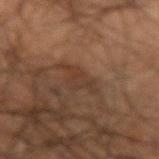Clinical impression:
Part of a total-body skin-imaging series; this lesion was reviewed on a skin check and was not flagged for biopsy.
Background:
The lesion's longest dimension is about 4.5 mm. An algorithmic analysis of the crop reported an outline eccentricity of about 0.85 (0 = round, 1 = elongated). The analysis additionally found a border-irregularity rating of about 7/10 and internal color variation of about 2.5 on a 0–10 scale. The software also gave a classifier nevus-likeness of about 0/100 and a detector confidence of about 50 out of 100 that the crop contains a lesion. From the left forearm. Captured under cross-polarized illumination. A male subject roughly 70 years of age. A lesion tile, about 15 mm wide, cut from a 3D total-body photograph.